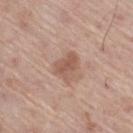Approximately 3.5 mm at its widest. A male patient about 70 years old. Located on the right thigh. Captured under white-light illumination. A lesion tile, about 15 mm wide, cut from a 3D total-body photograph.